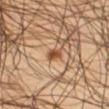The lesion was tiled from a total-body skin photograph and was not biopsied. Captured under cross-polarized illumination. From the leg. The subject is a male roughly 45 years of age. Approximately 2 mm at its widest. A lesion tile, about 15 mm wide, cut from a 3D total-body photograph.